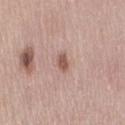Impression: Part of a total-body skin-imaging series; this lesion was reviewed on a skin check and was not flagged for biopsy. Context: Located on the abdomen. The subject is a female aged 48–52. A close-up tile cropped from a whole-body skin photograph, about 15 mm across. The recorded lesion diameter is about 2.5 mm.